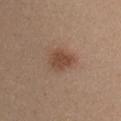Imaged during a routine full-body skin examination; the lesion was not biopsied and no histopathology is available. An algorithmic analysis of the crop reported an area of roughly 6 mm², an outline eccentricity of about 0.6 (0 = round, 1 = elongated), and a symmetry-axis asymmetry near 0.3. On the chest. Cropped from a total-body skin-imaging series; the visible field is about 15 mm. Measured at roughly 3 mm in maximum diameter. Captured under white-light illumination. A female subject in their mid- to late 40s.